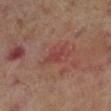The lesion was tiled from a total-body skin photograph and was not biopsied. From the leg. A male patient, aged 68–72. A region of skin cropped from a whole-body photographic capture, roughly 15 mm wide. Measured at roughly 3 mm in maximum diameter.